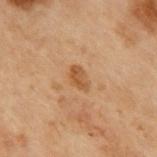Q: Is there a histopathology result?
A: catalogued during a skin exam; not biopsied
Q: What did automated image analysis measure?
A: a lesion area of about 3.5 mm² and a shape-asymmetry score of about 0.3 (0 = symmetric); roughly 8 lightness units darker than nearby skin and a normalized border contrast of about 7; a border-irregularity index near 3/10, a color-variation rating of about 1.5/10, and radial color variation of about 0.5
Q: What is the imaging modality?
A: ~15 mm tile from a whole-body skin photo
Q: How was the tile lit?
A: cross-polarized illumination
Q: Where on the body is the lesion?
A: the back
Q: What are the patient's age and sex?
A: male, approximately 55 years of age